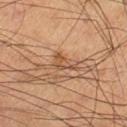Impression:
This lesion was catalogued during total-body skin photography and was not selected for biopsy.
Clinical summary:
The subject is a male approximately 60 years of age. A close-up tile cropped from a whole-body skin photograph, about 15 mm across. About 3.5 mm across. The lesion is on the right lower leg. Imaged with cross-polarized lighting.